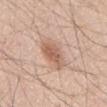| field | value |
|---|---|
| follow-up | catalogued during a skin exam; not biopsied |
| patient | male, aged 43 to 47 |
| image source | total-body-photography crop, ~15 mm field of view |
| anatomic site | the abdomen |